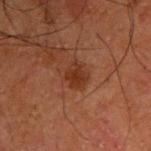| feature | finding |
|---|---|
| biopsy status | catalogued during a skin exam; not biopsied |
| image source | ~15 mm crop, total-body skin-cancer survey |
| patient | male, roughly 50 years of age |
| anatomic site | the upper back |